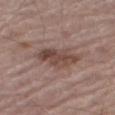Q: How large is the lesion?
A: ~5.5 mm (longest diameter)
Q: How was this image acquired?
A: ~15 mm tile from a whole-body skin photo
Q: Who is the patient?
A: male, aged around 80
Q: Automated lesion metrics?
A: a mean CIELAB color near L≈45 a*≈18 b*≈23, roughly 11 lightness units darker than nearby skin, and a normalized border contrast of about 8
Q: Where on the body is the lesion?
A: the left leg
Q: How was the tile lit?
A: white-light